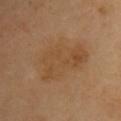| field | value |
|---|---|
| follow-up | no biopsy performed (imaged during a skin exam) |
| image source | ~15 mm tile from a whole-body skin photo |
| diameter | ~7 mm (longest diameter) |
| subject | female, about 60 years old |
| anatomic site | the arm |